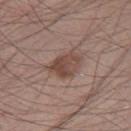notes — total-body-photography surveillance lesion; no biopsy | automated metrics — a footprint of about 9.5 mm² and an eccentricity of roughly 0.75; a mean CIELAB color near L≈46 a*≈18 b*≈23 and roughly 10 lightness units darker than nearby skin; a border-irregularity rating of about 3/10, a within-lesion color-variation index near 4.5/10, and a peripheral color-asymmetry measure near 1.5; an automated nevus-likeness rating near 35 out of 100 and a lesion-detection confidence of about 100/100 | subject — male, roughly 45 years of age | site — the right thigh | acquisition — total-body-photography crop, ~15 mm field of view.